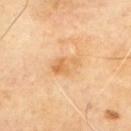Q: Was this lesion biopsied?
A: no biopsy performed (imaged during a skin exam)
Q: What is the anatomic site?
A: the upper back
Q: Who is the patient?
A: male, in their mid-60s
Q: What kind of image is this?
A: 15 mm crop, total-body photography
Q: What is the lesion's diameter?
A: about 3.5 mm
Q: How was the tile lit?
A: cross-polarized